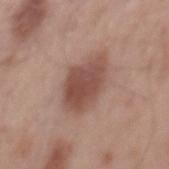Assessment: The lesion was photographed on a routine skin check and not biopsied; there is no pathology result. Image and clinical context: On the mid back. A lesion tile, about 15 mm wide, cut from a 3D total-body photograph. A male subject aged approximately 55. The lesion's longest dimension is about 6 mm.